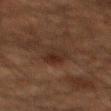• notes — total-body-photography surveillance lesion; no biopsy
• acquisition — 15 mm crop, total-body photography
• patient — male, aged 58 to 62
• site — the back